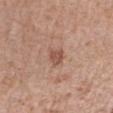follow-up — total-body-photography surveillance lesion; no biopsy | imaging modality — ~15 mm tile from a whole-body skin photo | automated metrics — a border-irregularity rating of about 2/10 and radial color variation of about 0.5 | anatomic site — the arm | illumination — white-light | patient — female, in their 60s | diameter — about 2.5 mm.